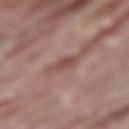Impression: Recorded during total-body skin imaging; not selected for excision or biopsy. Clinical summary: This is a white-light tile. The lesion is located on the left thigh. A 15 mm close-up extracted from a 3D total-body photography capture. Automated image analysis of the tile measured a footprint of about 4 mm² and an outline eccentricity of about 0.75 (0 = round, 1 = elongated). The analysis additionally found a classifier nevus-likeness of about 0/100. The subject is a male aged around 40.